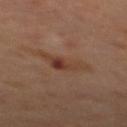Impression: Imaged during a routine full-body skin examination; the lesion was not biopsied and no histopathology is available. Background: A male subject aged around 70. A roughly 15 mm field-of-view crop from a total-body skin photograph. Longest diameter approximately 5 mm. The total-body-photography lesion software estimated an outline eccentricity of about 0.9 (0 = round, 1 = elongated) and a shape-asymmetry score of about 0.5 (0 = symmetric). It also reported an automated nevus-likeness rating near 0 out of 100 and lesion-presence confidence of about 100/100. Captured under cross-polarized illumination. The lesion is on the mid back.